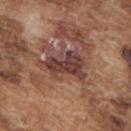Q: Was this lesion biopsied?
A: no biopsy performed (imaged during a skin exam)
Q: How was this image acquired?
A: ~15 mm tile from a whole-body skin photo
Q: What did automated image analysis measure?
A: about 12 CIELAB-L* units darker than the surrounding skin and a normalized lesion–skin contrast near 10.5
Q: Where on the body is the lesion?
A: the upper back
Q: Patient demographics?
A: male, about 75 years old
Q: What lighting was used for the tile?
A: white-light